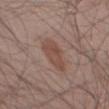This lesion was catalogued during total-body skin photography and was not selected for biopsy. The lesion is located on the left thigh. The subject is a male in their mid-50s. The lesion-visualizer software estimated a lesion color around L≈47 a*≈19 b*≈24 in CIELAB and a normalized border contrast of about 6.5. It also reported a classifier nevus-likeness of about 80/100 and lesion-presence confidence of about 100/100. This is a white-light tile. A close-up tile cropped from a whole-body skin photograph, about 15 mm across.